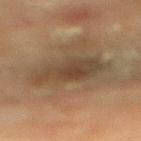biopsy_status: not biopsied; imaged during a skin examination
image:
  source: total-body photography crop
  field_of_view_mm: 15
patient:
  sex: female
  age_approx: 80
site: mid back